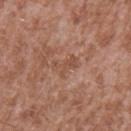biopsy_status: not biopsied; imaged during a skin examination
lighting: white-light
patient:
  sex: male
  age_approx: 45
automated_metrics:
  cielab_L: 50
  cielab_a: 22
  cielab_b: 29
  vs_skin_darker_L: 6.0
  vs_skin_contrast_norm: 5.0
  color_variation_0_10: 0.5
  peripheral_color_asymmetry: 0.0
lesion_size:
  long_diameter_mm_approx: 3.0
image:
  source: total-body photography crop
  field_of_view_mm: 15
site: left upper arm